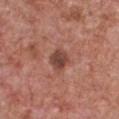Q: What is the lesion's diameter?
A: about 2.5 mm
Q: Where on the body is the lesion?
A: the chest
Q: Patient demographics?
A: male, aged 63–67
Q: Automated lesion metrics?
A: a footprint of about 4.5 mm², an eccentricity of roughly 0.55, and two-axis asymmetry of about 0.15; a mean CIELAB color near L≈43 a*≈24 b*≈25, about 12 CIELAB-L* units darker than the surrounding skin, and a normalized lesion–skin contrast near 9; an automated nevus-likeness rating near 15 out of 100 and a detector confidence of about 100 out of 100 that the crop contains a lesion
Q: What is the imaging modality?
A: ~15 mm crop, total-body skin-cancer survey
Q: Illumination type?
A: white-light illumination